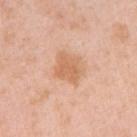Recorded during total-body skin imaging; not selected for excision or biopsy.